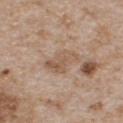Clinical impression: Imaged during a routine full-body skin examination; the lesion was not biopsied and no histopathology is available. Context: The lesion is located on the chest. A male patient, aged approximately 65. Captured under white-light illumination. The total-body-photography lesion software estimated an area of roughly 8 mm², a shape eccentricity near 0.85, and a symmetry-axis asymmetry near 0.35. And it measured an average lesion color of about L≈56 a*≈16 b*≈29 (CIELAB) and about 8 CIELAB-L* units darker than the surrounding skin. The software also gave an automated nevus-likeness rating near 0 out of 100 and a detector confidence of about 100 out of 100 that the crop contains a lesion. Approximately 4 mm at its widest. A 15 mm close-up tile from a total-body photography series done for melanoma screening.